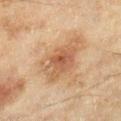| feature | finding |
|---|---|
| notes | catalogued during a skin exam; not biopsied |
| patient | female, aged 58–62 |
| body site | the right lower leg |
| image | total-body-photography crop, ~15 mm field of view |
| lighting | cross-polarized |